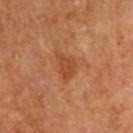follow-up = catalogued during a skin exam; not biopsied | imaging modality = ~15 mm tile from a whole-body skin photo | patient = male, aged around 65 | site = the right upper arm.